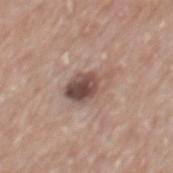The lesion was tiled from a total-body skin photograph and was not biopsied.
Located on the mid back.
Cropped from a whole-body photographic skin survey; the tile spans about 15 mm.
The patient is a male in their 70s.
Measured at roughly 4.5 mm in maximum diameter.